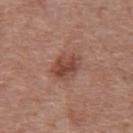Case summary:
- workup: catalogued during a skin exam; not biopsied
- subject: male, aged 73 to 77
- image-analysis metrics: an eccentricity of roughly 0.5 and two-axis asymmetry of about 0.15; roughly 10 lightness units darker than nearby skin; a border-irregularity index near 1.5/10, a color-variation rating of about 5/10, and radial color variation of about 1.5
- image: total-body-photography crop, ~15 mm field of view
- lesion size: about 3.5 mm
- body site: the abdomen
- lighting: white-light illumination